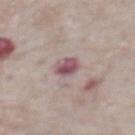The lesion was tiled from a total-body skin photograph and was not biopsied.
A male subject in their mid- to late 70s.
This is a white-light tile.
From the abdomen.
Longest diameter approximately 3 mm.
A lesion tile, about 15 mm wide, cut from a 3D total-body photograph.